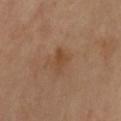Impression:
Part of a total-body skin-imaging series; this lesion was reviewed on a skin check and was not flagged for biopsy.
Image and clinical context:
The tile uses cross-polarized illumination. The lesion is on the leg. A female patient aged approximately 70. A roughly 15 mm field-of-view crop from a total-body skin photograph. The lesion's longest dimension is about 3 mm.